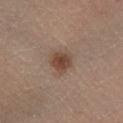Assessment: No biopsy was performed on this lesion — it was imaged during a full skin examination and was not determined to be concerning. Context: Automated image analysis of the tile measured an area of roughly 6 mm² and two-axis asymmetry of about 0.25. The software also gave internal color variation of about 3 on a 0–10 scale and a peripheral color-asymmetry measure near 1. And it measured an automated nevus-likeness rating near 95 out of 100. The patient is a female about 40 years old. Cropped from a whole-body photographic skin survey; the tile spans about 15 mm. The lesion is located on the left leg.